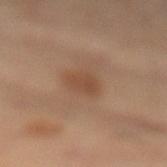Assessment: Recorded during total-body skin imaging; not selected for excision or biopsy. Background: Captured under cross-polarized illumination. A 15 mm close-up extracted from a 3D total-body photography capture. Located on the left leg. The patient is a male aged approximately 50.